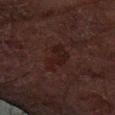biopsy_status: not biopsied; imaged during a skin examination
site: left forearm
image:
  source: total-body photography crop
  field_of_view_mm: 15
lesion_size:
  long_diameter_mm_approx: 4.5
patient:
  sex: male
  age_approx: 70
lighting: cross-polarized